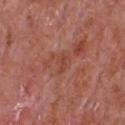Impression: This lesion was catalogued during total-body skin photography and was not selected for biopsy. Image and clinical context: Cropped from a total-body skin-imaging series; the visible field is about 15 mm. The subject is a male aged around 65. The total-body-photography lesion software estimated an area of roughly 3.5 mm², an eccentricity of roughly 0.85, and two-axis asymmetry of about 0.3. And it measured a lesion color around L≈46 a*≈26 b*≈30 in CIELAB and about 5 CIELAB-L* units darker than the surrounding skin. The software also gave border irregularity of about 3.5 on a 0–10 scale, a within-lesion color-variation index near 1/10, and peripheral color asymmetry of about 0.5. It also reported a classifier nevus-likeness of about 0/100 and lesion-presence confidence of about 85/100. The lesion's longest dimension is about 3 mm. On the front of the torso. Captured under white-light illumination.